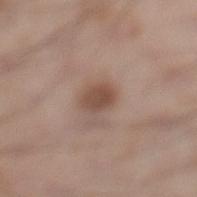Impression: This lesion was catalogued during total-body skin photography and was not selected for biopsy. Clinical summary: This is a white-light tile. A region of skin cropped from a whole-body photographic capture, roughly 15 mm wide. The patient is a male approximately 35 years of age. The lesion is located on the left lower leg. Automated image analysis of the tile measured a lesion color around L≈49 a*≈18 b*≈26 in CIELAB and a lesion-to-skin contrast of about 8 (normalized; higher = more distinct). The software also gave a border-irregularity index near 1.5/10, a within-lesion color-variation index near 2/10, and peripheral color asymmetry of about 0.5. The software also gave a classifier nevus-likeness of about 90/100 and lesion-presence confidence of about 100/100.